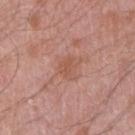This lesion was catalogued during total-body skin photography and was not selected for biopsy. A male patient, roughly 50 years of age. A lesion tile, about 15 mm wide, cut from a 3D total-body photograph. Located on the right upper arm.